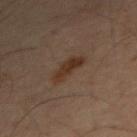Impression:
Imaged during a routine full-body skin examination; the lesion was not biopsied and no histopathology is available.
Image and clinical context:
The lesion is located on the left arm. About 3.5 mm across. A male patient, aged 48 to 52. This is a cross-polarized tile. A close-up tile cropped from a whole-body skin photograph, about 15 mm across.